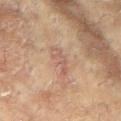{"image": {"source": "total-body photography crop", "field_of_view_mm": 15}, "site": "right arm", "patient": {"sex": "female", "age_approx": 80}, "automated_metrics": {"eccentricity": 0.9, "shape_asymmetry": 0.4, "cielab_L": 52, "cielab_a": 18, "cielab_b": 26, "vs_skin_darker_L": 6.0, "vs_skin_contrast_norm": 5.0, "color_variation_0_10": 1.0}}